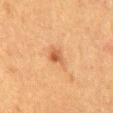notes: catalogued during a skin exam; not biopsied
subject: male, in their 50s
TBP lesion metrics: a lesion color around L≈48 a*≈23 b*≈34 in CIELAB, a lesion–skin lightness drop of about 9, and a lesion-to-skin contrast of about 7 (normalized; higher = more distinct); a within-lesion color-variation index near 3.5/10 and peripheral color asymmetry of about 1.5; an automated nevus-likeness rating near 70 out of 100 and lesion-presence confidence of about 100/100
image: ~15 mm tile from a whole-body skin photo
body site: the mid back
tile lighting: cross-polarized
size: ≈3 mm